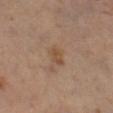  biopsy_status: not biopsied; imaged during a skin examination
  lesion_size:
    long_diameter_mm_approx: 2.5
  image:
    source: total-body photography crop
    field_of_view_mm: 15
  site: leg
  patient:
    sex: male
    age_approx: 60
  lighting: cross-polarized